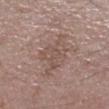Findings:
– follow-up — no biopsy performed (imaged during a skin exam)
– image source — 15 mm crop, total-body photography
– size — ≈6 mm
– tile lighting — white-light illumination
– automated metrics — two-axis asymmetry of about 0.4; an average lesion color of about L≈51 a*≈16 b*≈23 (CIELAB), a lesion–skin lightness drop of about 7, and a lesion-to-skin contrast of about 5.5 (normalized; higher = more distinct); border irregularity of about 5.5 on a 0–10 scale, a color-variation rating of about 2.5/10, and radial color variation of about 1; an automated nevus-likeness rating near 0 out of 100 and a lesion-detection confidence of about 100/100
– patient — male, about 60 years old
– body site — the leg